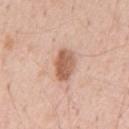Impression:
This lesion was catalogued during total-body skin photography and was not selected for biopsy.
Acquisition and patient details:
Measured at roughly 4 mm in maximum diameter. Automated image analysis of the tile measured a lesion color around L≈60 a*≈22 b*≈31 in CIELAB, about 13 CIELAB-L* units darker than the surrounding skin, and a normalized lesion–skin contrast near 8.5. The analysis additionally found an automated nevus-likeness rating near 85 out of 100 and a lesion-detection confidence of about 100/100. This image is a 15 mm lesion crop taken from a total-body photograph. The lesion is on the front of the torso. A male subject, aged approximately 55. Captured under white-light illumination.